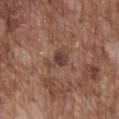  biopsy_status: not biopsied; imaged during a skin examination
  site: chest
  lighting: white-light
  patient:
    sex: male
    age_approx: 75
  image:
    source: total-body photography crop
    field_of_view_mm: 15
  automated_metrics:
    eccentricity: 0.7
  lesion_size:
    long_diameter_mm_approx: 3.0